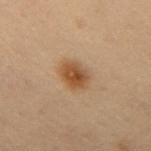biopsy_status: not biopsied; imaged during a skin examination
site: mid back
lighting: cross-polarized
image:
  source: total-body photography crop
  field_of_view_mm: 15
patient:
  sex: male
  age_approx: 55
lesion_size:
  long_diameter_mm_approx: 3.5